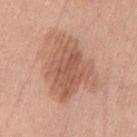follow-up = no biopsy performed (imaged during a skin exam) | lesion size = ≈8 mm | tile lighting = white-light | image source = ~15 mm crop, total-body skin-cancer survey | automated lesion analysis = a lesion area of about 32 mm², a shape eccentricity near 0.7, and a symmetry-axis asymmetry near 0.4; a lesion color around L≈58 a*≈22 b*≈30 in CIELAB and a normalized border contrast of about 7; a classifier nevus-likeness of about 5/100 | patient = female, in their mid-60s | anatomic site = the leg.